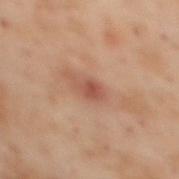Captured during whole-body skin photography for melanoma surveillance; the lesion was not biopsied.
The recorded lesion diameter is about 3 mm.
The subject is a female aged 53–57.
From the upper back.
A 15 mm close-up tile from a total-body photography series done for melanoma screening.
An algorithmic analysis of the crop reported a footprint of about 4.5 mm², an eccentricity of roughly 0.85, and two-axis asymmetry of about 0.25. The software also gave an average lesion color of about L≈43 a*≈20 b*≈25 (CIELAB), roughly 8 lightness units darker than nearby skin, and a normalized border contrast of about 6.5.
Imaged with cross-polarized lighting.